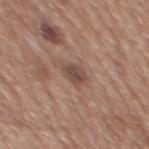Q: Is there a histopathology result?
A: imaged on a skin check; not biopsied
Q: Where on the body is the lesion?
A: the mid back
Q: What did automated image analysis measure?
A: a lesion area of about 4 mm², a shape eccentricity near 0.65, and two-axis asymmetry of about 0.25
Q: What kind of image is this?
A: ~15 mm crop, total-body skin-cancer survey
Q: Illumination type?
A: white-light illumination
Q: How large is the lesion?
A: ~2.5 mm (longest diameter)
Q: Who is the patient?
A: male, aged 63 to 67